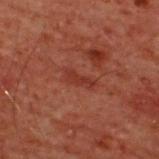<lesion>
<biopsy_status>not biopsied; imaged during a skin examination</biopsy_status>
<patient>
  <sex>male</sex>
  <age_approx>60</age_approx>
</patient>
<site>upper back</site>
<image>
  <source>total-body photography crop</source>
  <field_of_view_mm>15</field_of_view_mm>
</image>
<automated_metrics>
  <border_irregularity_0_10>3.5</border_irregularity_0_10>
  <color_variation_0_10>0.0</color_variation_0_10>
  <peripheral_color_asymmetry>0.0</peripheral_color_asymmetry>
</automated_metrics>
<lighting>cross-polarized</lighting>
</lesion>